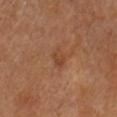Q: Is there a histopathology result?
A: total-body-photography surveillance lesion; no biopsy
Q: What is the lesion's diameter?
A: ~2.5 mm (longest diameter)
Q: What is the imaging modality?
A: total-body-photography crop, ~15 mm field of view
Q: Illumination type?
A: cross-polarized illumination
Q: What is the anatomic site?
A: the head or neck
Q: What are the patient's age and sex?
A: female, approximately 55 years of age